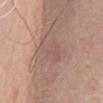<record>
  <biopsy_status>not biopsied; imaged during a skin examination</biopsy_status>
  <lighting>white-light</lighting>
  <site>chest</site>
  <image>
    <source>total-body photography crop</source>
    <field_of_view_mm>15</field_of_view_mm>
  </image>
  <patient>
    <sex>male</sex>
    <age_approx>60</age_approx>
  </patient>
</record>